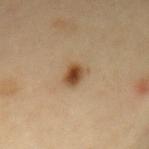biopsy_status: not biopsied; imaged during a skin examination
automated_metrics:
  eccentricity: 0.65
  shape_asymmetry: 0.25
  vs_skin_contrast_norm: 10.0
lesion_size:
  long_diameter_mm_approx: 3.0
patient:
  sex: female
  age_approx: 55
site: abdomen
lighting: cross-polarized
image:
  source: total-body photography crop
  field_of_view_mm: 15